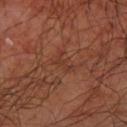Case summary:
* workup: no biopsy performed (imaged during a skin exam)
* imaging modality: ~15 mm tile from a whole-body skin photo
* anatomic site: the arm
* diameter: ≈3 mm
* tile lighting: cross-polarized
* patient: male, approximately 65 years of age
* automated lesion analysis: a mean CIELAB color near L≈36 a*≈23 b*≈29, a lesion–skin lightness drop of about 5, and a normalized lesion–skin contrast near 4.5; internal color variation of about 0 on a 0–10 scale and a peripheral color-asymmetry measure near 0; a classifier nevus-likeness of about 0/100 and a detector confidence of about 95 out of 100 that the crop contains a lesion